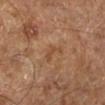A subject aged approximately 65. The lesion is located on the left lower leg. Imaged with cross-polarized lighting. A 15 mm close-up extracted from a 3D total-body photography capture.